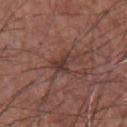<case>
  <biopsy_status>not biopsied; imaged during a skin examination</biopsy_status>
  <automated_metrics>
    <area_mm2_approx>5.5</area_mm2_approx>
    <nevus_likeness_0_100>5</nevus_likeness_0_100>
    <lesion_detection_confidence_0_100>85</lesion_detection_confidence_0_100>
  </automated_metrics>
  <site>front of the torso</site>
  <image>
    <source>total-body photography crop</source>
    <field_of_view_mm>15</field_of_view_mm>
  </image>
  <patient>
    <sex>male</sex>
    <age_approx>75</age_approx>
  </patient>
  <lighting>white-light</lighting>
  <lesion_size>
    <long_diameter_mm_approx>4.0</long_diameter_mm_approx>
  </lesion_size>
</case>